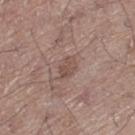  biopsy_status: not biopsied; imaged during a skin examination
  patient:
    sex: male
    age_approx: 65
  automated_metrics:
    eccentricity: 0.8
    shape_asymmetry: 0.25
    cielab_L: 49
    cielab_a: 17
    cielab_b: 23
    vs_skin_darker_L: 8.0
    vs_skin_contrast_norm: 6.0
    nevus_likeness_0_100: 0
    lesion_detection_confidence_0_100: 100
  image:
    source: total-body photography crop
    field_of_view_mm: 15
  site: left thigh
  lesion_size:
    long_diameter_mm_approx: 3.0